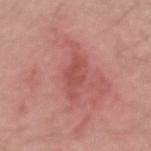Context: The patient is a male approximately 55 years of age. The total-body-photography lesion software estimated a lesion area of about 7 mm², an outline eccentricity of about 0.95 (0 = round, 1 = elongated), and a symmetry-axis asymmetry near 0.3. It also reported a classifier nevus-likeness of about 0/100. The lesion is located on the left forearm. This is a white-light tile. The recorded lesion diameter is about 5 mm. A close-up tile cropped from a whole-body skin photograph, about 15 mm across.